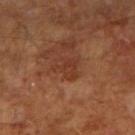Assessment:
Part of a total-body skin-imaging series; this lesion was reviewed on a skin check and was not flagged for biopsy.
Image and clinical context:
The tile uses cross-polarized illumination. A male patient about 65 years old. The total-body-photography lesion software estimated a footprint of about 6 mm², an eccentricity of roughly 0.5, and two-axis asymmetry of about 0.5. The software also gave a border-irregularity index near 5/10 and a color-variation rating of about 2.5/10. It also reported lesion-presence confidence of about 100/100. A region of skin cropped from a whole-body photographic capture, roughly 15 mm wide. Measured at roughly 3.5 mm in maximum diameter. The lesion is on the right upper arm.